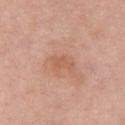  biopsy_status: not biopsied; imaged during a skin examination
  patient:
    sex: female
    age_approx: 70
  lesion_size:
    long_diameter_mm_approx: 2.5
  site: chest
  automated_metrics:
    cielab_L: 58
    cielab_a: 24
    cielab_b: 32
    vs_skin_darker_L: 6.0
    vs_skin_contrast_norm: 5.0
  lighting: white-light
  image:
    source: total-body photography crop
    field_of_view_mm: 15